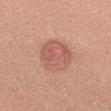Clinical impression:
No biopsy was performed on this lesion — it was imaged during a full skin examination and was not determined to be concerning.